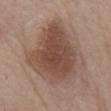biopsy status: imaged on a skin check; not biopsied | anatomic site: the chest | subject: male, approximately 85 years of age or older | image: total-body-photography crop, ~15 mm field of view | lighting: white-light | lesion diameter: about 8.5 mm.